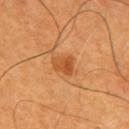size: ~2.5 mm (longest diameter)
TBP lesion metrics: a shape eccentricity near 0.7; border irregularity of about 2.5 on a 0–10 scale; a nevus-likeness score of about 95/100 and a detector confidence of about 100 out of 100 that the crop contains a lesion
location: the mid back
imaging modality: ~15 mm tile from a whole-body skin photo
illumination: cross-polarized
patient: male, aged around 60